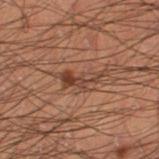subject = male, in their mid- to late 50s
tile lighting = cross-polarized illumination
image source = ~15 mm tile from a whole-body skin photo
automated lesion analysis = an automated nevus-likeness rating near 20 out of 100
anatomic site = the right thigh
lesion diameter = ~4.5 mm (longest diameter)